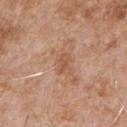The tile uses white-light illumination. The lesion's longest dimension is about 2.5 mm. Automated image analysis of the tile measured a lesion–skin lightness drop of about 7 and a normalized lesion–skin contrast near 5.5. The analysis additionally found an automated nevus-likeness rating near 0 out of 100 and a lesion-detection confidence of about 100/100. On the front of the torso. A male patient, in their mid-60s. A lesion tile, about 15 mm wide, cut from a 3D total-body photograph.